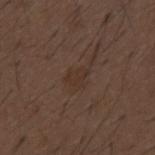The lesion is on the back.
A 15 mm close-up extracted from a 3D total-body photography capture.
A male patient aged approximately 50.